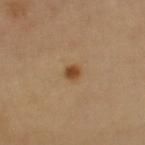Assessment: Part of a total-body skin-imaging series; this lesion was reviewed on a skin check and was not flagged for biopsy. Context: The lesion's longest dimension is about 2 mm. The tile uses cross-polarized illumination. The lesion is on the right upper arm. A roughly 15 mm field-of-view crop from a total-body skin photograph. A female subject about 65 years old. The lesion-visualizer software estimated a mean CIELAB color near L≈45 a*≈20 b*≈36 and roughly 11 lightness units darker than nearby skin. The analysis additionally found a detector confidence of about 100 out of 100 that the crop contains a lesion.